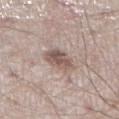This lesion was catalogued during total-body skin photography and was not selected for biopsy.
Captured under white-light illumination.
The patient is a male aged 58–62.
A close-up tile cropped from a whole-body skin photograph, about 15 mm across.
The lesion's longest dimension is about 4 mm.
On the leg.